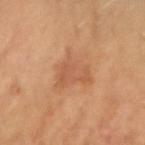This lesion was catalogued during total-body skin photography and was not selected for biopsy.
The patient is a female roughly 55 years of age.
Longest diameter approximately 5 mm.
Cropped from a total-body skin-imaging series; the visible field is about 15 mm.
The lesion is on the right forearm.